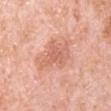Q: Was this lesion biopsied?
A: no biopsy performed (imaged during a skin exam)
Q: What lighting was used for the tile?
A: white-light illumination
Q: Who is the patient?
A: male, in their 80s
Q: Automated lesion metrics?
A: an area of roughly 12 mm², a shape eccentricity near 0.8, and a shape-asymmetry score of about 0.4 (0 = symmetric); an average lesion color of about L≈65 a*≈26 b*≈32 (CIELAB) and a lesion–skin lightness drop of about 9; lesion-presence confidence of about 100/100
Q: Lesion location?
A: the left upper arm
Q: How was this image acquired?
A: total-body-photography crop, ~15 mm field of view
Q: How large is the lesion?
A: about 5 mm